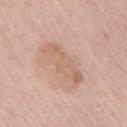biopsy_status: not biopsied; imaged during a skin examination
lesion_size:
  long_diameter_mm_approx: 6.0
lighting: white-light
patient:
  sex: male
  age_approx: 55
site: arm
image:
  source: total-body photography crop
  field_of_view_mm: 15